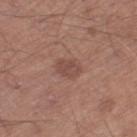follow-up: total-body-photography surveillance lesion; no biopsy | site: the left thigh | illumination: white-light illumination | subject: male, aged 63–67 | lesion size: ≈3 mm | automated metrics: border irregularity of about 2 on a 0–10 scale, a color-variation rating of about 2.5/10, and peripheral color asymmetry of about 1; a classifier nevus-likeness of about 10/100 and a detector confidence of about 100 out of 100 that the crop contains a lesion | acquisition: total-body-photography crop, ~15 mm field of view.